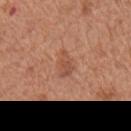Part of a total-body skin-imaging series; this lesion was reviewed on a skin check and was not flagged for biopsy.
Captured under white-light illumination.
A male patient roughly 65 years of age.
The lesion is located on the mid back.
A 15 mm close-up tile from a total-body photography series done for melanoma screening.
The lesion's longest dimension is about 3.5 mm.